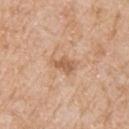notes: no biopsy performed (imaged during a skin exam)
tile lighting: white-light
site: the chest
subject: male, roughly 65 years of age
acquisition: 15 mm crop, total-body photography
size: about 3 mm